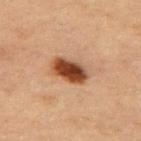diameter: ≈4.5 mm | anatomic site: the leg | patient: female, in their mid- to late 50s | imaging modality: ~15 mm crop, total-body skin-cancer survey.